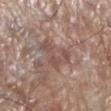workup: no biopsy performed (imaged during a skin exam) | size: ≈4 mm | automated lesion analysis: a lesion area of about 7 mm² and two-axis asymmetry of about 0.75; an average lesion color of about L≈50 a*≈18 b*≈23 (CIELAB), about 8 CIELAB-L* units darker than the surrounding skin, and a normalized lesion–skin contrast near 6; a nevus-likeness score of about 0/100 | image: ~15 mm crop, total-body skin-cancer survey | patient: male, aged around 70 | location: the left lower leg.